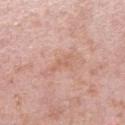Q: What are the patient's age and sex?
A: male, aged 38 to 42
Q: Where on the body is the lesion?
A: the right upper arm
Q: What is the imaging modality?
A: ~15 mm crop, total-body skin-cancer survey